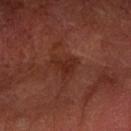Q: Was this lesion biopsied?
A: total-body-photography surveillance lesion; no biopsy
Q: Lesion location?
A: the arm
Q: How was the tile lit?
A: cross-polarized
Q: Patient demographics?
A: male, aged around 65
Q: How large is the lesion?
A: ≈3 mm
Q: What is the imaging modality?
A: ~15 mm tile from a whole-body skin photo